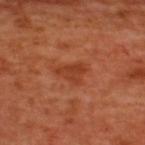{"biopsy_status": "not biopsied; imaged during a skin examination", "site": "back", "image": {"source": "total-body photography crop", "field_of_view_mm": 15}, "automated_metrics": {"cielab_L": 41, "cielab_a": 30, "cielab_b": 36, "vs_skin_darker_L": 8.0, "border_irregularity_0_10": 5.0, "color_variation_0_10": 2.0, "peripheral_color_asymmetry": 0.5, "lesion_detection_confidence_0_100": 100}, "lesion_size": {"long_diameter_mm_approx": 3.5}, "patient": {"sex": "male", "age_approx": 50}, "lighting": "cross-polarized"}